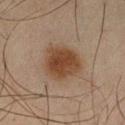* biopsy status — imaged on a skin check; not biopsied
* anatomic site — the left thigh
* lighting — cross-polarized illumination
* lesion size — ≈4.5 mm
* patient — male, aged 43–47
* image source — ~15 mm tile from a whole-body skin photo